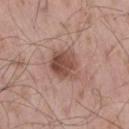Assessment: Recorded during total-body skin imaging; not selected for excision or biopsy. Context: The lesion is located on the right thigh. A male subject, approximately 55 years of age. Captured under white-light illumination. A 15 mm close-up tile from a total-body photography series done for melanoma screening. The recorded lesion diameter is about 3.5 mm.